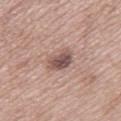Findings:
– follow-up: catalogued during a skin exam; not biopsied
– subject: female, aged 53 to 57
– lighting: white-light
– body site: the right thigh
– diameter: about 3 mm
– image source: total-body-photography crop, ~15 mm field of view